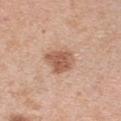biopsy status=catalogued during a skin exam; not biopsied
acquisition=~15 mm tile from a whole-body skin photo
size=~3.5 mm (longest diameter)
patient=female, aged 38 to 42
anatomic site=the arm